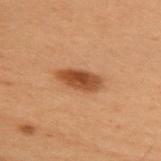Clinical impression: Part of a total-body skin-imaging series; this lesion was reviewed on a skin check and was not flagged for biopsy. Clinical summary: From the upper back. A female subject, about 50 years old. A 15 mm crop from a total-body photograph taken for skin-cancer surveillance. The lesion's longest dimension is about 4.5 mm.